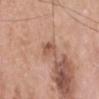Recorded during total-body skin imaging; not selected for excision or biopsy.
Automated image analysis of the tile measured an outline eccentricity of about 0.85 (0 = round, 1 = elongated) and two-axis asymmetry of about 0.25. It also reported a mean CIELAB color near L≈55 a*≈21 b*≈29 and a normalized border contrast of about 6.5.
The lesion is on the head or neck.
Longest diameter approximately 3 mm.
This is a white-light tile.
A male subject in their mid- to late 60s.
A region of skin cropped from a whole-body photographic capture, roughly 15 mm wide.